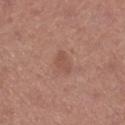Findings:
- workup · catalogued during a skin exam; not biopsied
- illumination · white-light illumination
- diameter · ~2.5 mm (longest diameter)
- automated lesion analysis · a footprint of about 3 mm², a shape eccentricity near 0.8, and a symmetry-axis asymmetry near 0.45; a border-irregularity rating of about 4/10, a within-lesion color-variation index near 1.5/10, and peripheral color asymmetry of about 0.5; a nevus-likeness score of about 0/100
- patient · female, approximately 50 years of age
- anatomic site · the left lower leg
- image source · ~15 mm tile from a whole-body skin photo